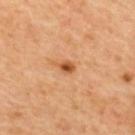Clinical impression: Imaged during a routine full-body skin examination; the lesion was not biopsied and no histopathology is available. Context: Longest diameter approximately 2 mm. Captured under cross-polarized illumination. Cropped from a total-body skin-imaging series; the visible field is about 15 mm. The lesion is located on the mid back. The subject is a male aged approximately 60. The lesion-visualizer software estimated a lesion area of about 2.5 mm², an outline eccentricity of about 0.7 (0 = round, 1 = elongated), and two-axis asymmetry of about 0.3. It also reported a border-irregularity index near 2.5/10 and radial color variation of about 1. And it measured a nevus-likeness score of about 90/100 and a detector confidence of about 100 out of 100 that the crop contains a lesion.